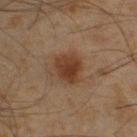Q: Was this lesion biopsied?
A: no biopsy performed (imaged during a skin exam)
Q: Illumination type?
A: cross-polarized illumination
Q: Lesion size?
A: ≈4 mm
Q: Who is the patient?
A: male, in their mid- to late 40s
Q: Automated lesion metrics?
A: a lesion color around L≈30 a*≈16 b*≈24 in CIELAB, about 8 CIELAB-L* units darker than the surrounding skin, and a normalized lesion–skin contrast near 8.5; peripheral color asymmetry of about 1; a classifier nevus-likeness of about 95/100 and lesion-presence confidence of about 100/100
Q: What is the anatomic site?
A: the left lower leg
Q: What is the imaging modality?
A: 15 mm crop, total-body photography